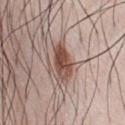Q: Was a biopsy performed?
A: total-body-photography surveillance lesion; no biopsy
Q: What is the imaging modality?
A: 15 mm crop, total-body photography
Q: What did automated image analysis measure?
A: an outline eccentricity of about 0.9 (0 = round, 1 = elongated); a mean CIELAB color near L≈49 a*≈20 b*≈25
Q: Where on the body is the lesion?
A: the front of the torso
Q: What are the patient's age and sex?
A: male, in their mid- to late 30s
Q: Lesion size?
A: about 5 mm